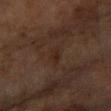Clinical impression:
Captured during whole-body skin photography for melanoma surveillance; the lesion was not biopsied.